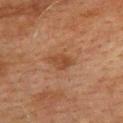biopsy status = imaged on a skin check; not biopsied | subject = male, aged 73–77 | anatomic site = the upper back | size = about 3.5 mm | lighting = cross-polarized | image = ~15 mm crop, total-body skin-cancer survey.